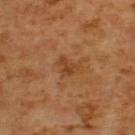Imaged during a routine full-body skin examination; the lesion was not biopsied and no histopathology is available.
This is a cross-polarized tile.
The lesion is on the upper back.
A 15 mm close-up extracted from a 3D total-body photography capture.
A male subject, aged around 65.
Measured at roughly 2.5 mm in maximum diameter.
The total-body-photography lesion software estimated a border-irregularity index near 4.5/10, a within-lesion color-variation index near 1.5/10, and a peripheral color-asymmetry measure near 0.5. The analysis additionally found a nevus-likeness score of about 0/100 and a lesion-detection confidence of about 100/100.